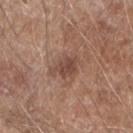{"site": "right forearm", "image": {"source": "total-body photography crop", "field_of_view_mm": 15}, "automated_metrics": {"cielab_L": 47, "cielab_a": 19, "cielab_b": 25, "vs_skin_darker_L": 9.0, "vs_skin_contrast_norm": 7.0, "border_irregularity_0_10": 4.0, "nevus_likeness_0_100": 10, "lesion_detection_confidence_0_100": 100}, "lighting": "white-light", "lesion_size": {"long_diameter_mm_approx": 4.0}, "patient": {"sex": "male", "age_approx": 60}}